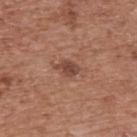| field | value |
|---|---|
| notes | total-body-photography surveillance lesion; no biopsy |
| image source | total-body-photography crop, ~15 mm field of view |
| subject | male, about 75 years old |
| location | the upper back |